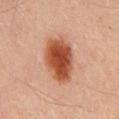Q: Was this lesion biopsied?
A: no biopsy performed (imaged during a skin exam)
Q: Patient demographics?
A: male, about 45 years old
Q: Lesion location?
A: the mid back
Q: How was the tile lit?
A: cross-polarized
Q: What did automated image analysis measure?
A: a mean CIELAB color near L≈41 a*≈23 b*≈29, a lesion–skin lightness drop of about 13, and a normalized lesion–skin contrast near 11; border irregularity of about 1.5 on a 0–10 scale, internal color variation of about 5.5 on a 0–10 scale, and radial color variation of about 1.5
Q: What is the imaging modality?
A: total-body-photography crop, ~15 mm field of view